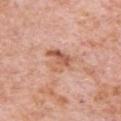Impression:
This lesion was catalogued during total-body skin photography and was not selected for biopsy.
Image and clinical context:
The lesion is located on the chest. Automated image analysis of the tile measured a lesion color around L≈60 a*≈24 b*≈32 in CIELAB and about 10 CIELAB-L* units darker than the surrounding skin. It also reported a border-irregularity index near 4/10 and a within-lesion color-variation index near 8/10. The analysis additionally found a lesion-detection confidence of about 100/100. A male patient, about 80 years old. Measured at roughly 4 mm in maximum diameter. Captured under white-light illumination. A close-up tile cropped from a whole-body skin photograph, about 15 mm across.